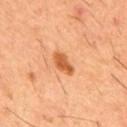This lesion was catalogued during total-body skin photography and was not selected for biopsy. Measured at roughly 3.5 mm in maximum diameter. A 15 mm close-up extracted from a 3D total-body photography capture. An algorithmic analysis of the crop reported an area of roughly 4.5 mm², an outline eccentricity of about 0.85 (0 = round, 1 = elongated), and a symmetry-axis asymmetry near 0.3. The analysis additionally found an average lesion color of about L≈55 a*≈28 b*≈42 (CIELAB), about 12 CIELAB-L* units darker than the surrounding skin, and a normalized border contrast of about 8.5. And it measured a border-irregularity rating of about 3/10, a within-lesion color-variation index near 2/10, and radial color variation of about 0.5. A male subject, approximately 60 years of age. On the back.